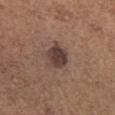Assessment:
Imaged during a routine full-body skin examination; the lesion was not biopsied and no histopathology is available.
Background:
The recorded lesion diameter is about 3.5 mm. A 15 mm close-up extracted from a 3D total-body photography capture. The patient is a male in their mid-60s. Imaged with white-light lighting. The lesion is on the head or neck.